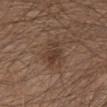Q: Was this lesion biopsied?
A: no biopsy performed (imaged during a skin exam)
Q: What did automated image analysis measure?
A: an outline eccentricity of about 0.7 (0 = round, 1 = elongated); border irregularity of about 3.5 on a 0–10 scale and a peripheral color-asymmetry measure near 1; a nevus-likeness score of about 10/100 and a detector confidence of about 100 out of 100 that the crop contains a lesion
Q: Lesion location?
A: the abdomen
Q: Who is the patient?
A: male, in their mid- to late 60s
Q: What kind of image is this?
A: total-body-photography crop, ~15 mm field of view